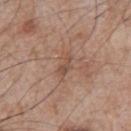No biopsy was performed on this lesion — it was imaged during a full skin examination and was not determined to be concerning. From the left upper arm. Captured under white-light illumination. A male patient, aged 63–67. A 15 mm close-up extracted from a 3D total-body photography capture. The lesion's longest dimension is about 2.5 mm.